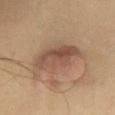anatomic site — the chest
illumination — cross-polarized
image-analysis metrics — a border-irregularity index near 2.5/10, a color-variation rating of about 6/10, and a peripheral color-asymmetry measure near 2.5; a nevus-likeness score of about 75/100 and a lesion-detection confidence of about 100/100
acquisition — ~15 mm crop, total-body skin-cancer survey
patient — male, about 55 years old